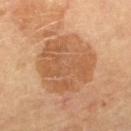Q: Is there a histopathology result?
A: total-body-photography surveillance lesion; no biopsy
Q: What is the imaging modality?
A: total-body-photography crop, ~15 mm field of view
Q: What is the anatomic site?
A: the left thigh
Q: Patient demographics?
A: male, aged 58 to 62